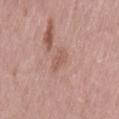| key | value |
|---|---|
| lesion diameter | ~3 mm (longest diameter) |
| TBP lesion metrics | an area of roughly 4 mm², a shape eccentricity near 0.85, and a shape-asymmetry score of about 0.3 (0 = symmetric); about 7 CIELAB-L* units darker than the surrounding skin and a lesion-to-skin contrast of about 5 (normalized; higher = more distinct); a border-irregularity rating of about 3/10 and internal color variation of about 1.5 on a 0–10 scale; a classifier nevus-likeness of about 0/100 and a detector confidence of about 100 out of 100 that the crop contains a lesion |
| subject | female, aged around 40 |
| location | the lower back |
| illumination | white-light illumination |
| imaging modality | ~15 mm tile from a whole-body skin photo |